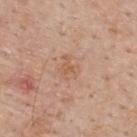The lesion was photographed on a routine skin check and not biopsied; there is no pathology result.
The recorded lesion diameter is about 3 mm.
The patient is a male approximately 60 years of age.
Imaged with white-light lighting.
A lesion tile, about 15 mm wide, cut from a 3D total-body photograph.
Automated tile analysis of the lesion measured a lesion area of about 5 mm². The software also gave a mean CIELAB color near L≈59 a*≈21 b*≈32, roughly 6 lightness units darker than nearby skin, and a normalized border contrast of about 5. The software also gave a nevus-likeness score of about 0/100 and a detector confidence of about 100 out of 100 that the crop contains a lesion.
On the upper back.